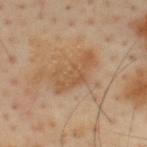follow-up=catalogued during a skin exam; not biopsied | size=≈5 mm | anatomic site=the upper back | patient=male, aged around 55 | imaging modality=~15 mm crop, total-body skin-cancer survey | lighting=cross-polarized | image-analysis metrics=an eccentricity of roughly 0.85 and two-axis asymmetry of about 0.4; an average lesion color of about L≈54 a*≈19 b*≈35 (CIELAB) and a normalized lesion–skin contrast near 6; a detector confidence of about 100 out of 100 that the crop contains a lesion.